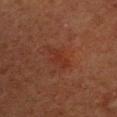Impression:
Imaged during a routine full-body skin examination; the lesion was not biopsied and no histopathology is available.
Clinical summary:
A region of skin cropped from a whole-body photographic capture, roughly 15 mm wide. The patient is a female about 40 years old. Measured at roughly 3 mm in maximum diameter. Located on the chest. The total-body-photography lesion software estimated an area of roughly 4 mm². It also reported a lesion color around L≈28 a*≈23 b*≈27 in CIELAB and a lesion-to-skin contrast of about 5 (normalized; higher = more distinct). It also reported a border-irregularity rating of about 5/10, a color-variation rating of about 1/10, and radial color variation of about 0.5. The analysis additionally found a detector confidence of about 100 out of 100 that the crop contains a lesion. This is a cross-polarized tile.